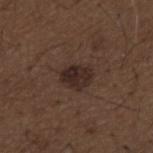Clinical summary: A male patient, aged approximately 50. Cropped from a whole-body photographic skin survey; the tile spans about 15 mm. About 3.5 mm across. The tile uses white-light illumination. On the mid back.